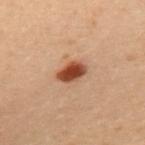The lesion's longest dimension is about 3.5 mm. A female subject, in their 30s. A roughly 15 mm field-of-view crop from a total-body skin photograph. This is a cross-polarized tile. The lesion is located on the upper back.